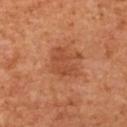follow-up: imaged on a skin check; not biopsied.